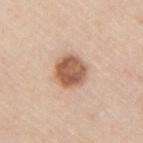diameter — ≈3.5 mm; illumination — white-light; acquisition — 15 mm crop, total-body photography; patient — male, in their mid-40s; body site — the right upper arm.